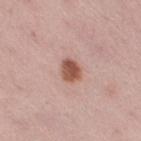Imaged during a routine full-body skin examination; the lesion was not biopsied and no histopathology is available. A female subject, in their mid- to late 30s. A 15 mm crop from a total-body photograph taken for skin-cancer surveillance. On the right thigh.